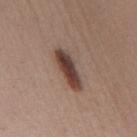Impression:
Recorded during total-body skin imaging; not selected for excision or biopsy.
Acquisition and patient details:
This is a white-light tile. A male patient aged 38–42. A 15 mm close-up tile from a total-body photography series done for melanoma screening. The lesion is on the mid back.